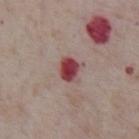follow-up = catalogued during a skin exam; not biopsied | image-analysis metrics = border irregularity of about 2 on a 0–10 scale and a peripheral color-asymmetry measure near 1.5 | image source = ~15 mm crop, total-body skin-cancer survey | body site = the chest | tile lighting = white-light illumination | size = ~3 mm (longest diameter) | subject = male, aged 73 to 77.